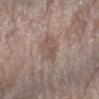biopsy_status: not biopsied; imaged during a skin examination
lighting: white-light
site: arm
automated_metrics:
  cielab_L: 53
  cielab_a: 15
  cielab_b: 23
  vs_skin_contrast_norm: 5.0
  border_irregularity_0_10: 2.5
  color_variation_0_10: 3.0
  peripheral_color_asymmetry: 1.0
  nevus_likeness_0_100: 15
  lesion_detection_confidence_0_100: 100
patient:
  sex: male
  age_approx: 80
image:
  source: total-body photography crop
  field_of_view_mm: 15